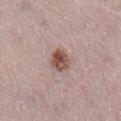biopsy status = total-body-photography surveillance lesion; no biopsy | diameter = ~2.5 mm (longest diameter) | image = total-body-photography crop, ~15 mm field of view | automated metrics = a within-lesion color-variation index near 6/10; an automated nevus-likeness rating near 95 out of 100 and a lesion-detection confidence of about 100/100 | lighting = white-light illumination | subject = female, about 50 years old | anatomic site = the leg.